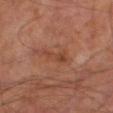Q: Was this lesion biopsied?
A: total-body-photography surveillance lesion; no biopsy
Q: How was this image acquired?
A: ~15 mm crop, total-body skin-cancer survey
Q: What did automated image analysis measure?
A: internal color variation of about 1.5 on a 0–10 scale and radial color variation of about 0.5; a detector confidence of about 100 out of 100 that the crop contains a lesion
Q: What is the anatomic site?
A: the right leg
Q: What is the lesion's diameter?
A: ~3.5 mm (longest diameter)
Q: Who is the patient?
A: male, aged approximately 60